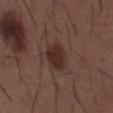- lesion size · ~4 mm (longest diameter)
- TBP lesion metrics · an automated nevus-likeness rating near 90 out of 100 and lesion-presence confidence of about 100/100
- tile lighting · white-light illumination
- location · the mid back
- subject · male, aged approximately 50
- acquisition · ~15 mm crop, total-body skin-cancer survey